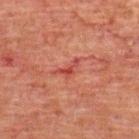Impression: Recorded during total-body skin imaging; not selected for excision or biopsy.